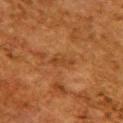follow-up: total-body-photography surveillance lesion; no biopsy
image: ~15 mm tile from a whole-body skin photo
subject: female, roughly 50 years of age
lesion diameter: about 3.5 mm
site: the upper back